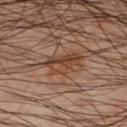Captured during whole-body skin photography for melanoma surveillance; the lesion was not biopsied.